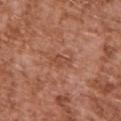Impression:
Captured during whole-body skin photography for melanoma surveillance; the lesion was not biopsied.
Context:
A male subject aged around 75. On the upper back. A 15 mm crop from a total-body photograph taken for skin-cancer surveillance.